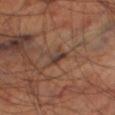biopsy_status: not biopsied; imaged during a skin examination
site: leg
lighting: cross-polarized
patient:
  sex: male
  age_approx: 70
image:
  source: total-body photography crop
  field_of_view_mm: 15
lesion_size:
  long_diameter_mm_approx: 2.5